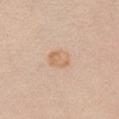Q: Was a biopsy performed?
A: catalogued during a skin exam; not biopsied
Q: How large is the lesion?
A: ≈3 mm
Q: What did automated image analysis measure?
A: a lesion color around L≈67 a*≈18 b*≈33 in CIELAB, a lesion–skin lightness drop of about 7, and a normalized lesion–skin contrast near 6; an automated nevus-likeness rating near 55 out of 100 and a detector confidence of about 100 out of 100 that the crop contains a lesion
Q: Patient demographics?
A: female, approximately 25 years of age
Q: How was this image acquired?
A: ~15 mm tile from a whole-body skin photo
Q: How was the tile lit?
A: white-light illumination
Q: Lesion location?
A: the right upper arm